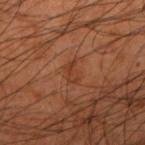Q: What is the anatomic site?
A: the right upper arm
Q: How was the tile lit?
A: cross-polarized illumination
Q: What kind of image is this?
A: ~15 mm tile from a whole-body skin photo
Q: Lesion size?
A: ~2.5 mm (longest diameter)
Q: Patient demographics?
A: male, roughly 45 years of age